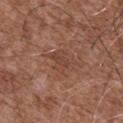The lesion was photographed on a routine skin check and not biopsied; there is no pathology result. The patient is a male approximately 75 years of age. A roughly 15 mm field-of-view crop from a total-body skin photograph. Located on the chest. This is a white-light tile.